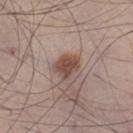| key | value |
|---|---|
| notes | total-body-photography surveillance lesion; no biopsy |
| subject | male, in their mid-40s |
| diameter | ~3.5 mm (longest diameter) |
| image source | ~15 mm crop, total-body skin-cancer survey |
| body site | the left thigh |
| tile lighting | white-light illumination |